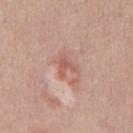Background:
On the abdomen. This is a white-light tile. Longest diameter approximately 2.5 mm. Cropped from a whole-body photographic skin survey; the tile spans about 15 mm. The patient is a male roughly 40 years of age.